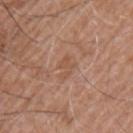The lesion was photographed on a routine skin check and not biopsied; there is no pathology result. From the left upper arm. The subject is a male in their mid-70s. Cropped from a whole-body photographic skin survey; the tile spans about 15 mm.